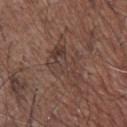Q: Is there a histopathology result?
A: total-body-photography surveillance lesion; no biopsy
Q: How large is the lesion?
A: ≈6.5 mm
Q: Automated lesion metrics?
A: a lesion area of about 12 mm², an outline eccentricity of about 0.85 (0 = round, 1 = elongated), and two-axis asymmetry of about 0.6; an automated nevus-likeness rating near 0 out of 100 and a detector confidence of about 70 out of 100 that the crop contains a lesion
Q: What is the anatomic site?
A: the front of the torso
Q: Patient demographics?
A: male, approximately 70 years of age
Q: How was this image acquired?
A: 15 mm crop, total-body photography
Q: How was the tile lit?
A: white-light